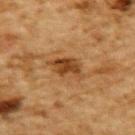Findings:
* follow-up: no biopsy performed (imaged during a skin exam)
* anatomic site: the upper back
* patient: male, aged 83 or over
* automated metrics: internal color variation of about 3.5 on a 0–10 scale and radial color variation of about 1
* acquisition: total-body-photography crop, ~15 mm field of view
* illumination: cross-polarized
* size: about 4 mm